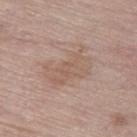  biopsy_status: not biopsied; imaged during a skin examination
  lighting: white-light
  patient:
    sex: male
    age_approx: 80
  automated_metrics:
    eccentricity: 0.8
    shape_asymmetry: 0.25
    cielab_L: 58
    cielab_a: 16
    cielab_b: 26
    vs_skin_darker_L: 6.0
    border_irregularity_0_10: 5.0
    color_variation_0_10: 2.5
    nevus_likeness_0_100: 0
    lesion_detection_confidence_0_100: 100
  image:
    source: total-body photography crop
    field_of_view_mm: 15
  site: left thigh
  lesion_size:
    long_diameter_mm_approx: 5.5